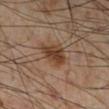A close-up tile cropped from a whole-body skin photograph, about 15 mm across. Located on the right lower leg. A male patient, about 55 years old. Measured at roughly 4 mm in maximum diameter. Imaged with cross-polarized lighting. Automated tile analysis of the lesion measured a lesion color around L≈40 a*≈19 b*≈30 in CIELAB and a normalized lesion–skin contrast near 10. It also reported border irregularity of about 2.5 on a 0–10 scale and a peripheral color-asymmetry measure near 1.